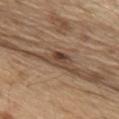The lesion was tiled from a total-body skin photograph and was not biopsied. Located on the back. The tile uses white-light illumination. A region of skin cropped from a whole-body photographic capture, roughly 15 mm wide. A male subject aged around 70. Automated image analysis of the tile measured a lesion area of about 4.5 mm² and two-axis asymmetry of about 0.2. The software also gave roughly 11 lightness units darker than nearby skin and a lesion-to-skin contrast of about 8.5 (normalized; higher = more distinct). It also reported border irregularity of about 2 on a 0–10 scale and a color-variation rating of about 7.5/10. The software also gave a classifier nevus-likeness of about 20/100 and a detector confidence of about 100 out of 100 that the crop contains a lesion. Approximately 3 mm at its widest.